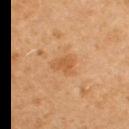Q: Lesion location?
A: the left upper arm
Q: What is the lesion's diameter?
A: about 3 mm
Q: What kind of image is this?
A: total-body-photography crop, ~15 mm field of view
Q: Patient demographics?
A: female, aged around 50